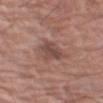Impression: This lesion was catalogued during total-body skin photography and was not selected for biopsy.